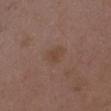{
  "biopsy_status": "not biopsied; imaged during a skin examination",
  "image": {
    "source": "total-body photography crop",
    "field_of_view_mm": 15
  },
  "site": "chest",
  "patient": {
    "sex": "female",
    "age_approx": 35
  },
  "lesion_size": {
    "long_diameter_mm_approx": 3.0
  },
  "lighting": "white-light"
}